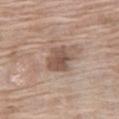Case summary:
- biopsy status · no biopsy performed (imaged during a skin exam)
- acquisition · 15 mm crop, total-body photography
- diameter · ~3.5 mm (longest diameter)
- subject · female, roughly 75 years of age
- site · the right thigh
- TBP lesion metrics · a lesion color around L≈52 a*≈16 b*≈25 in CIELAB, about 11 CIELAB-L* units darker than the surrounding skin, and a normalized border contrast of about 8; a border-irregularity index near 2.5/10, a color-variation rating of about 3/10, and peripheral color asymmetry of about 1
- illumination · white-light